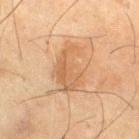follow-up = imaged on a skin check; not biopsied
site = the right thigh
patient = male, aged 63–67
lesion diameter = ~5.5 mm (longest diameter)
imaging modality = ~15 mm crop, total-body skin-cancer survey
lighting = cross-polarized illumination
automated lesion analysis = a classifier nevus-likeness of about 10/100 and a detector confidence of about 100 out of 100 that the crop contains a lesion